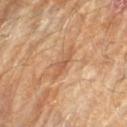This lesion was catalogued during total-body skin photography and was not selected for biopsy.
About 2.5 mm across.
A male patient, in their mid-80s or older.
The lesion is on the left forearm.
A roughly 15 mm field-of-view crop from a total-body skin photograph.
This is a cross-polarized tile.